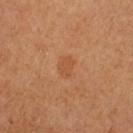biopsy status = total-body-photography surveillance lesion; no biopsy | lesion size = ~2.5 mm (longest diameter) | acquisition = 15 mm crop, total-body photography | anatomic site = the left upper arm | patient = male, aged 58–62 | illumination = cross-polarized illumination.